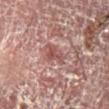{
  "biopsy_status": "not biopsied; imaged during a skin examination",
  "lesion_size": {
    "long_diameter_mm_approx": 3.0
  },
  "automated_metrics": {
    "cielab_L": 45,
    "cielab_a": 21,
    "cielab_b": 21,
    "vs_skin_contrast_norm": 6.5,
    "border_irregularity_0_10": 3.5,
    "peripheral_color_asymmetry": 1.0,
    "lesion_detection_confidence_0_100": 90
  },
  "lighting": "cross-polarized",
  "patient": {
    "sex": "male",
    "age_approx": 55
  },
  "site": "leg",
  "image": {
    "source": "total-body photography crop",
    "field_of_view_mm": 15
  }
}